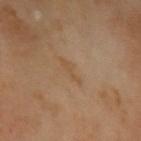Background:
Located on the left upper arm. This image is a 15 mm lesion crop taken from a total-body photograph. A male subject aged approximately 70. Approximately 3.5 mm at its widest.